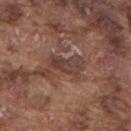Part of a total-body skin-imaging series; this lesion was reviewed on a skin check and was not flagged for biopsy. Longest diameter approximately 4 mm. A male subject aged around 75. Located on the upper back. This is a white-light tile. Cropped from a total-body skin-imaging series; the visible field is about 15 mm.